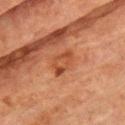workup — catalogued during a skin exam; not biopsied
subject — male, about 75 years old
anatomic site — the chest
diameter — about 3.5 mm
image — 15 mm crop, total-body photography